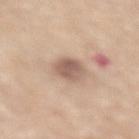Background: On the lower back. A 15 mm crop from a total-body photograph taken for skin-cancer surveillance. A male patient, aged 68–72. Captured under white-light illumination.